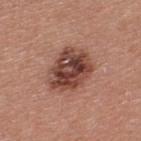The lesion was tiled from a total-body skin photograph and was not biopsied. The tile uses white-light illumination. Longest diameter approximately 5.5 mm. The subject is a male approximately 40 years of age. A close-up tile cropped from a whole-body skin photograph, about 15 mm across. Located on the upper back.